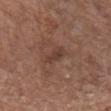| feature | finding |
|---|---|
| biopsy status | catalogued during a skin exam; not biopsied |
| TBP lesion metrics | an average lesion color of about L≈39 a*≈19 b*≈24 (CIELAB) and roughly 7 lightness units darker than nearby skin; border irregularity of about 2.5 on a 0–10 scale and peripheral color asymmetry of about 0.5 |
| anatomic site | the chest |
| imaging modality | ~15 mm crop, total-body skin-cancer survey |
| patient | male, in their 80s |
| tile lighting | white-light |